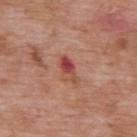Impression: Part of a total-body skin-imaging series; this lesion was reviewed on a skin check and was not flagged for biopsy. Clinical summary: About 3.5 mm across. The total-body-photography lesion software estimated a footprint of about 4 mm² and an eccentricity of roughly 0.85. It also reported an average lesion color of about L≈47 a*≈32 b*≈27 (CIELAB), roughly 11 lightness units darker than nearby skin, and a normalized border contrast of about 8.5. And it measured a within-lesion color-variation index near 2.5/10 and radial color variation of about 0.5. The software also gave lesion-presence confidence of about 100/100. The patient is a male aged around 60. The lesion is on the upper back. A close-up tile cropped from a whole-body skin photograph, about 15 mm across. Captured under white-light illumination.